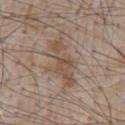Part of a total-body skin-imaging series; this lesion was reviewed on a skin check and was not flagged for biopsy. Located on the chest. A region of skin cropped from a whole-body photographic capture, roughly 15 mm wide. Automated image analysis of the tile measured an area of roughly 13 mm², a shape eccentricity near 0.9, and a symmetry-axis asymmetry near 0.4. And it measured a normalized border contrast of about 6. It also reported a border-irregularity rating of about 6/10, a within-lesion color-variation index near 3/10, and a peripheral color-asymmetry measure near 1. Imaged with white-light lighting. A male subject, aged approximately 65. Approximately 6 mm at its widest.